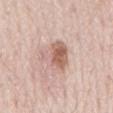Clinical impression: This lesion was catalogued during total-body skin photography and was not selected for biopsy. Acquisition and patient details: This is a white-light tile. The recorded lesion diameter is about 5 mm. Located on the mid back. A close-up tile cropped from a whole-body skin photograph, about 15 mm across. A male patient, aged around 80.